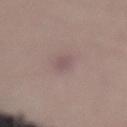Q: Is there a histopathology result?
A: no biopsy performed (imaged during a skin exam)
Q: Lesion size?
A: ~2.5 mm (longest diameter)
Q: Illumination type?
A: white-light illumination
Q: Where on the body is the lesion?
A: the lower back
Q: What kind of image is this?
A: ~15 mm crop, total-body skin-cancer survey
Q: What are the patient's age and sex?
A: female, in their mid-40s